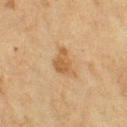Captured during whole-body skin photography for melanoma surveillance; the lesion was not biopsied. The lesion is on the left thigh. Imaged with cross-polarized lighting. The lesion-visualizer software estimated an area of roughly 6 mm². It also reported a lesion color around L≈48 a*≈17 b*≈35 in CIELAB, a lesion–skin lightness drop of about 8, and a normalized lesion–skin contrast near 7. The software also gave a border-irregularity rating of about 4/10, internal color variation of about 2 on a 0–10 scale, and peripheral color asymmetry of about 0.5. The software also gave a classifier nevus-likeness of about 20/100. A close-up tile cropped from a whole-body skin photograph, about 15 mm across. Measured at roughly 3.5 mm in maximum diameter. A female patient, roughly 55 years of age.